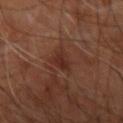No biopsy was performed on this lesion — it was imaged during a full skin examination and was not determined to be concerning. A roughly 15 mm field-of-view crop from a total-body skin photograph. Measured at roughly 3 mm in maximum diameter. From the right upper arm. A subject in their mid-60s. Captured under cross-polarized illumination. The lesion-visualizer software estimated a footprint of about 4.5 mm², a shape eccentricity near 0.65, and two-axis asymmetry of about 0.4. And it measured a mean CIELAB color near L≈28 a*≈22 b*≈24 and a lesion–skin lightness drop of about 7. The software also gave a lesion-detection confidence of about 100/100.